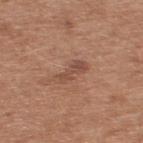follow-up=total-body-photography surveillance lesion; no biopsy
patient=male, about 55 years old
size=≈4 mm
site=the upper back
acquisition=~15 mm tile from a whole-body skin photo